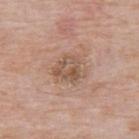Notes:
* workup · imaged on a skin check; not biopsied
* patient · male, aged approximately 75
* image · ~15 mm tile from a whole-body skin photo
* anatomic site · the upper back
* lesion size · about 4 mm
* automated lesion analysis · a normalized lesion–skin contrast near 6; border irregularity of about 3.5 on a 0–10 scale, a within-lesion color-variation index near 6/10, and peripheral color asymmetry of about 2; a lesion-detection confidence of about 100/100